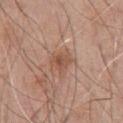An algorithmic analysis of the crop reported a footprint of about 5 mm² and an eccentricity of roughly 0.6. And it measured a classifier nevus-likeness of about 20/100 and a lesion-detection confidence of about 100/100. The lesion is on the chest. The tile uses white-light illumination. A 15 mm close-up tile from a total-body photography series done for melanoma screening. A male subject, aged approximately 70. Longest diameter approximately 2.5 mm.